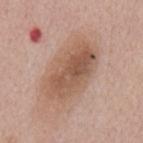Findings:
• site: the back
• tile lighting: white-light illumination
• lesion size: about 7.5 mm
• image: ~15 mm crop, total-body skin-cancer survey
• patient: male, aged 53 to 57